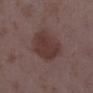The lesion was photographed on a routine skin check and not biopsied; there is no pathology result.
Imaged with white-light lighting.
A close-up tile cropped from a whole-body skin photograph, about 15 mm across.
Approximately 5 mm at its widest.
On the right lower leg.
The patient is a female about 35 years old.